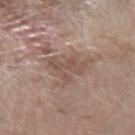| field | value |
|---|---|
| follow-up | no biopsy performed (imaged during a skin exam) |
| lesion diameter | ~5 mm (longest diameter) |
| acquisition | ~15 mm tile from a whole-body skin photo |
| automated lesion analysis | a lesion-to-skin contrast of about 6 (normalized; higher = more distinct); border irregularity of about 8 on a 0–10 scale and peripheral color asymmetry of about 1; an automated nevus-likeness rating near 0 out of 100 and a detector confidence of about 75 out of 100 that the crop contains a lesion |
| tile lighting | white-light illumination |
| body site | the left forearm |
| patient | male, roughly 80 years of age |